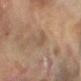– follow-up — catalogued during a skin exam; not biopsied
– diameter — ~2.5 mm (longest diameter)
– patient — female, roughly 80 years of age
– acquisition — ~15 mm tile from a whole-body skin photo
– body site — the right forearm
– lighting — cross-polarized illumination